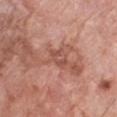Notes:
- workup — imaged on a skin check; not biopsied
- patient — male, aged 78 to 82
- site — the chest
- image source — ~15 mm tile from a whole-body skin photo
- TBP lesion metrics — a footprint of about 3 mm², an eccentricity of roughly 0.85, and two-axis asymmetry of about 0.45; a border-irregularity index near 6/10 and peripheral color asymmetry of about 0
- tile lighting — white-light illumination
- size — about 3 mm